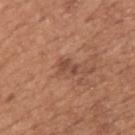• notes · no biopsy performed (imaged during a skin exam)
• location · the upper back
• image · ~15 mm tile from a whole-body skin photo
• subject · male, in their mid- to late 60s